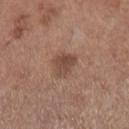Clinical impression: This lesion was catalogued during total-body skin photography and was not selected for biopsy. Acquisition and patient details: A female patient, roughly 55 years of age. The lesion is located on the left lower leg. This is a white-light tile. The recorded lesion diameter is about 3 mm. Cropped from a whole-body photographic skin survey; the tile spans about 15 mm.